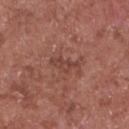Q: Is there a histopathology result?
A: no biopsy performed (imaged during a skin exam)
Q: Lesion location?
A: the front of the torso
Q: How was this image acquired?
A: ~15 mm tile from a whole-body skin photo
Q: Who is the patient?
A: male, aged 63 to 67
Q: How was the tile lit?
A: white-light illumination
Q: Automated lesion metrics?
A: a symmetry-axis asymmetry near 0.5; a border-irregularity index near 5/10, a within-lesion color-variation index near 2/10, and peripheral color asymmetry of about 1; an automated nevus-likeness rating near 0 out of 100
Q: How large is the lesion?
A: ≈3.5 mm